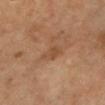Notes:
• image · 15 mm crop, total-body photography
• TBP lesion metrics · a footprint of about 4.5 mm² and a shape eccentricity near 0.85; roughly 6 lightness units darker than nearby skin and a normalized lesion–skin contrast near 5.5; lesion-presence confidence of about 100/100
• lighting · cross-polarized illumination
• lesion diameter · ~3.5 mm (longest diameter)
• site · the front of the torso
• patient · male, aged 53 to 57